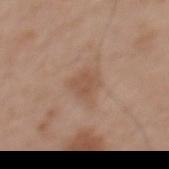No biopsy was performed on this lesion — it was imaged during a full skin examination and was not determined to be concerning.